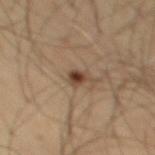| feature | finding |
|---|---|
| image | 15 mm crop, total-body photography |
| body site | the mid back |
| lesion diameter | ≈2.5 mm |
| tile lighting | cross-polarized illumination |
| subject | male, aged around 65 |